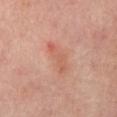Imaged during a routine full-body skin examination; the lesion was not biopsied and no histopathology is available.
A roughly 15 mm field-of-view crop from a total-body skin photograph.
On the mid back.
The recorded lesion diameter is about 4.5 mm.
The subject is a male aged approximately 65.